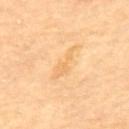{"biopsy_status": "not biopsied; imaged during a skin examination", "lesion_size": {"long_diameter_mm_approx": 5.0}, "image": {"source": "total-body photography crop", "field_of_view_mm": 15}, "lighting": "cross-polarized", "patient": {"sex": "male", "age_approx": "85+"}, "site": "upper back"}